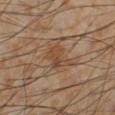workup=total-body-photography surveillance lesion; no biopsy | anatomic site=the left lower leg | image=15 mm crop, total-body photography | patient=male, about 55 years old.